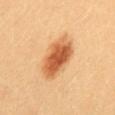Assessment:
The lesion was tiled from a total-body skin photograph and was not biopsied.
Acquisition and patient details:
A 15 mm close-up tile from a total-body photography series done for melanoma screening. From the mid back. The lesion-visualizer software estimated an area of roughly 15 mm², a shape eccentricity near 0.85, and a symmetry-axis asymmetry near 0.15. The analysis additionally found a border-irregularity index near 2/10, a color-variation rating of about 5.5/10, and a peripheral color-asymmetry measure near 1.5. It also reported a nevus-likeness score of about 100/100 and lesion-presence confidence of about 100/100. The patient is a female in their mid-20s. About 5.5 mm across. Imaged with cross-polarized lighting.